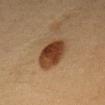| feature | finding |
|---|---|
| follow-up | no biopsy performed (imaged during a skin exam) |